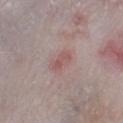Recorded during total-body skin imaging; not selected for excision or biopsy. The total-body-photography lesion software estimated a mean CIELAB color near L≈53 a*≈21 b*≈20, about 7 CIELAB-L* units darker than the surrounding skin, and a normalized lesion–skin contrast near 5.5. The patient is a male approximately 65 years of age. The tile uses white-light illumination. The lesion is located on the right lower leg. A lesion tile, about 15 mm wide, cut from a 3D total-body photograph. Longest diameter approximately 2.5 mm.